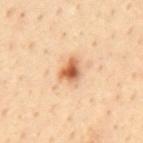biopsy status — total-body-photography surveillance lesion; no biopsy
diameter — about 3 mm
tile lighting — cross-polarized
automated lesion analysis — a lesion–skin lightness drop of about 17 and a normalized border contrast of about 10; a nevus-likeness score of about 95/100 and a lesion-detection confidence of about 100/100
image — ~15 mm tile from a whole-body skin photo
body site — the mid back
subject — male, aged 53–57